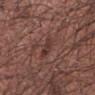Imaged during a routine full-body skin examination; the lesion was not biopsied and no histopathology is available.
A male subject aged around 55.
This image is a 15 mm lesion crop taken from a total-body photograph.
The lesion's longest dimension is about 3 mm.
The total-body-photography lesion software estimated an outline eccentricity of about 0.7 (0 = round, 1 = elongated). It also reported a nevus-likeness score of about 0/100 and lesion-presence confidence of about 100/100.
On the left forearm.